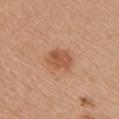Captured during whole-body skin photography for melanoma surveillance; the lesion was not biopsied. On the left upper arm. This image is a 15 mm lesion crop taken from a total-body photograph. A female subject aged 43–47.